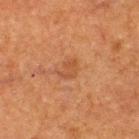Approximately 2.5 mm at its widest. Automated tile analysis of the lesion measured a lesion area of about 3.5 mm², a shape eccentricity near 0.75, and a symmetry-axis asymmetry near 0.35. It also reported a mean CIELAB color near L≈40 a*≈21 b*≈31, a lesion–skin lightness drop of about 6, and a normalized border contrast of about 5. And it measured a border-irregularity index near 3/10, internal color variation of about 2 on a 0–10 scale, and peripheral color asymmetry of about 0.5. The analysis additionally found a nevus-likeness score of about 10/100 and a detector confidence of about 100 out of 100 that the crop contains a lesion. The subject is a male in their 50s. Cropped from a whole-body photographic skin survey; the tile spans about 15 mm. The lesion is located on the back. This is a cross-polarized tile.